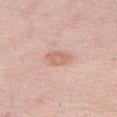Clinical impression:
The lesion was tiled from a total-body skin photograph and was not biopsied.
Image and clinical context:
A 15 mm crop from a total-body photograph taken for skin-cancer surveillance. A female patient roughly 50 years of age. The lesion is on the right thigh. The recorded lesion diameter is about 3.5 mm. Captured under white-light illumination.